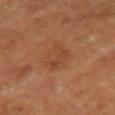Q: Was a biopsy performed?
A: total-body-photography surveillance lesion; no biopsy
Q: Lesion location?
A: the right forearm
Q: Lesion size?
A: about 3.5 mm
Q: What is the imaging modality?
A: ~15 mm tile from a whole-body skin photo
Q: Automated lesion metrics?
A: an area of roughly 4.5 mm², a shape eccentricity near 0.85, and two-axis asymmetry of about 0.65; a lesion color around L≈44 a*≈24 b*≈34 in CIELAB, a lesion–skin lightness drop of about 6, and a normalized border contrast of about 5; a within-lesion color-variation index near 1/10 and peripheral color asymmetry of about 0; a detector confidence of about 100 out of 100 that the crop contains a lesion
Q: Who is the patient?
A: female, aged 58–62